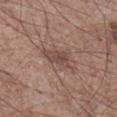Captured during whole-body skin photography for melanoma surveillance; the lesion was not biopsied.
The patient is a male aged 53 to 57.
A 15 mm crop from a total-body photograph taken for skin-cancer surveillance.
The lesion is located on the leg.
The total-body-photography lesion software estimated a nevus-likeness score of about 5/100.
Approximately 5 mm at its widest.
Imaged with white-light lighting.